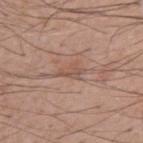<record>
<lighting>white-light</lighting>
<image>
  <source>total-body photography crop</source>
  <field_of_view_mm>15</field_of_view_mm>
</image>
<automated_metrics>
  <eccentricity>0.85</eccentricity>
  <shape_asymmetry>0.35</shape_asymmetry>
  <border_irregularity_0_10>4.5</border_irregularity_0_10>
  <color_variation_0_10>2.0</color_variation_0_10>
  <peripheral_color_asymmetry>0.5</peripheral_color_asymmetry>
</automated_metrics>
<patient>
  <sex>male</sex>
  <age_approx>55</age_approx>
</patient>
<lesion_size>
  <long_diameter_mm_approx>3.0</long_diameter_mm_approx>
</lesion_size>
</record>